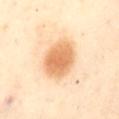Clinical impression:
Part of a total-body skin-imaging series; this lesion was reviewed on a skin check and was not flagged for biopsy.
Image and clinical context:
From the front of the torso. Approximately 5 mm at its widest. A female patient about 55 years old. This is a cross-polarized tile. A 15 mm close-up tile from a total-body photography series done for melanoma screening.